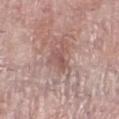Impression:
The lesion was photographed on a routine skin check and not biopsied; there is no pathology result.
Background:
A region of skin cropped from a whole-body photographic capture, roughly 15 mm wide. About 3 mm across. The lesion is located on the left lower leg. This is a white-light tile. A female subject, approximately 60 years of age.